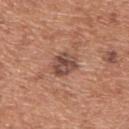This lesion was catalogued during total-body skin photography and was not selected for biopsy.
Approximately 3 mm at its widest.
A region of skin cropped from a whole-body photographic capture, roughly 15 mm wide.
Located on the upper back.
The patient is a male approximately 65 years of age.